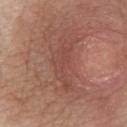Captured during whole-body skin photography for melanoma surveillance; the lesion was not biopsied.